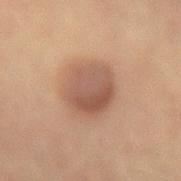biopsy status — imaged on a skin check; not biopsied
automated lesion analysis — a footprint of about 16 mm², an eccentricity of roughly 0.45, and two-axis asymmetry of about 0.1; a mean CIELAB color near L≈45 a*≈18 b*≈25, about 9 CIELAB-L* units darker than the surrounding skin, and a lesion-to-skin contrast of about 7 (normalized; higher = more distinct); border irregularity of about 1 on a 0–10 scale, a within-lesion color-variation index near 5/10, and radial color variation of about 2; an automated nevus-likeness rating near 95 out of 100
tile lighting — cross-polarized illumination
lesion diameter — ≈4.5 mm
image — ~15 mm tile from a whole-body skin photo
anatomic site — the left forearm
subject — female, roughly 65 years of age